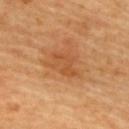| key | value |
|---|---|
| follow-up | catalogued during a skin exam; not biopsied |
| TBP lesion metrics | an area of roughly 8 mm² and an outline eccentricity of about 0.7 (0 = round, 1 = elongated); internal color variation of about 2.5 on a 0–10 scale; a nevus-likeness score of about 20/100 and a detector confidence of about 100 out of 100 that the crop contains a lesion |
| lesion size | about 4 mm |
| patient | female, aged 58 to 62 |
| tile lighting | cross-polarized |
| site | the back |
| acquisition | 15 mm crop, total-body photography |